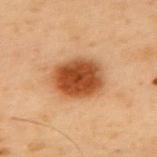Q: Is there a histopathology result?
A: imaged on a skin check; not biopsied
Q: Lesion size?
A: ≈5.5 mm
Q: What did automated image analysis measure?
A: an area of roughly 18 mm² and two-axis asymmetry of about 0.1; border irregularity of about 1 on a 0–10 scale and internal color variation of about 5 on a 0–10 scale; a classifier nevus-likeness of about 100/100 and a detector confidence of about 100 out of 100 that the crop contains a lesion
Q: What is the imaging modality?
A: total-body-photography crop, ~15 mm field of view
Q: What is the anatomic site?
A: the upper back
Q: Patient demographics?
A: male, roughly 55 years of age